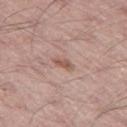| key | value |
|---|---|
| biopsy status | no biopsy performed (imaged during a skin exam) |
| image source | ~15 mm tile from a whole-body skin photo |
| site | the left thigh |
| patient | male, about 60 years old |